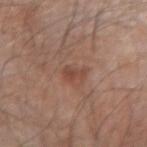follow-up: catalogued during a skin exam; not biopsied | illumination: white-light | anatomic site: the arm | imaging modality: 15 mm crop, total-body photography | diameter: ≈2.5 mm | subject: male, aged 68 to 72 | automated metrics: a footprint of about 3 mm²; a border-irregularity index near 4/10, internal color variation of about 0.5 on a 0–10 scale, and radial color variation of about 0; a detector confidence of about 100 out of 100 that the crop contains a lesion.